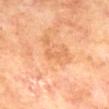Clinical impression:
Recorded during total-body skin imaging; not selected for excision or biopsy.
Acquisition and patient details:
A male patient, about 70 years old. The tile uses cross-polarized illumination. A lesion tile, about 15 mm wide, cut from a 3D total-body photograph. The lesion is on the back. The recorded lesion diameter is about 3 mm. Automated image analysis of the tile measured a lesion area of about 2.5 mm², an outline eccentricity of about 0.95 (0 = round, 1 = elongated), and a shape-asymmetry score of about 0.6 (0 = symmetric). And it measured border irregularity of about 7 on a 0–10 scale, internal color variation of about 0 on a 0–10 scale, and peripheral color asymmetry of about 0. And it measured a nevus-likeness score of about 0/100 and a lesion-detection confidence of about 100/100.